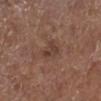The lesion was photographed on a routine skin check and not biopsied; there is no pathology result. Automated tile analysis of the lesion measured roughly 7 lightness units darker than nearby skin and a lesion-to-skin contrast of about 6.5 (normalized; higher = more distinct). It also reported border irregularity of about 3.5 on a 0–10 scale and internal color variation of about 1.5 on a 0–10 scale. It also reported a nevus-likeness score of about 5/100 and a lesion-detection confidence of about 100/100. A 15 mm close-up tile from a total-body photography series done for melanoma screening. This is a white-light tile. A male subject, in their mid- to late 70s. On the left lower leg.